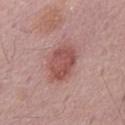Measured at roughly 5 mm in maximum diameter. The lesion is on the abdomen. An algorithmic analysis of the crop reported an area of roughly 13 mm², an outline eccentricity of about 0.7 (0 = round, 1 = elongated), and a shape-asymmetry score of about 0.15 (0 = symmetric). The analysis additionally found a classifier nevus-likeness of about 80/100 and lesion-presence confidence of about 100/100. A close-up tile cropped from a whole-body skin photograph, about 15 mm across. The subject is a male aged around 70.